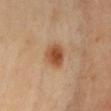{
  "patient": {
    "sex": "female",
    "age_approx": 40
  },
  "image": {
    "source": "total-body photography crop",
    "field_of_view_mm": 15
  },
  "lighting": "cross-polarized",
  "site": "chest"
}